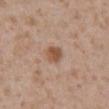Clinical impression: Imaged during a routine full-body skin examination; the lesion was not biopsied and no histopathology is available. Clinical summary: A 15 mm close-up extracted from a 3D total-body photography capture. Captured under white-light illumination. From the front of the torso. The patient is a male aged around 65. Measured at roughly 2.5 mm in maximum diameter.